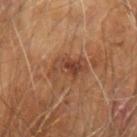| key | value |
|---|---|
| notes | catalogued during a skin exam; not biopsied |
| size | about 4.5 mm |
| illumination | cross-polarized |
| image | total-body-photography crop, ~15 mm field of view |
| location | the arm |
| subject | male, aged approximately 60 |